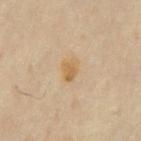Assessment: Imaged during a routine full-body skin examination; the lesion was not biopsied and no histopathology is available. Image and clinical context: A male patient aged 63 to 67. A region of skin cropped from a whole-body photographic capture, roughly 15 mm wide. Located on the chest. This is a cross-polarized tile. Longest diameter approximately 3 mm.